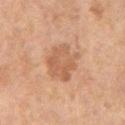Clinical impression:
No biopsy was performed on this lesion — it was imaged during a full skin examination and was not determined to be concerning.
Background:
A 15 mm close-up extracted from a 3D total-body photography capture. A female patient aged 58 to 62. Captured under cross-polarized illumination. On the left thigh. Automated image analysis of the tile measured a footprint of about 13 mm², an outline eccentricity of about 0.65 (0 = round, 1 = elongated), and a symmetry-axis asymmetry near 0.3. The software also gave border irregularity of about 3.5 on a 0–10 scale, internal color variation of about 3.5 on a 0–10 scale, and radial color variation of about 1. Approximately 5 mm at its widest.